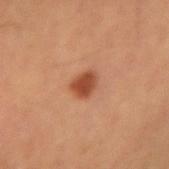site: arm
patient:
  sex: male
  age_approx: 60
image:
  source: total-body photography crop
  field_of_view_mm: 15